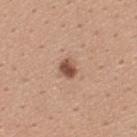Impression:
Captured during whole-body skin photography for melanoma surveillance; the lesion was not biopsied.